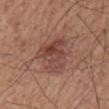Impression:
The lesion was tiled from a total-body skin photograph and was not biopsied.
Context:
Cropped from a whole-body photographic skin survey; the tile spans about 15 mm. A male patient in their mid-70s. Approximately 6 mm at its widest. On the back.